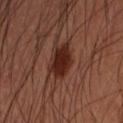The lesion was photographed on a routine skin check and not biopsied; there is no pathology result. Longest diameter approximately 4 mm. A 15 mm close-up tile from a total-body photography series done for melanoma screening. From the left forearm. A male patient roughly 50 years of age. Imaged with cross-polarized lighting. The total-body-photography lesion software estimated a lesion color around L≈25 a*≈23 b*≈25 in CIELAB, roughly 12 lightness units darker than nearby skin, and a normalized border contrast of about 12. The software also gave a nevus-likeness score of about 100/100 and lesion-presence confidence of about 100/100.